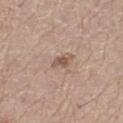Clinical impression:
Recorded during total-body skin imaging; not selected for excision or biopsy.
Clinical summary:
From the left thigh. The patient is a male approximately 65 years of age. This image is a 15 mm lesion crop taken from a total-body photograph. An algorithmic analysis of the crop reported a lesion area of about 3 mm² and two-axis asymmetry of about 0.25. The analysis additionally found a mean CIELAB color near L≈54 a*≈18 b*≈26 and roughly 11 lightness units darker than nearby skin. Imaged with white-light lighting. Longest diameter approximately 3 mm.